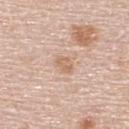A 15 mm close-up tile from a total-body photography series done for melanoma screening.
The lesion is on the upper back.
The lesion-visualizer software estimated radial color variation of about 0.5. The software also gave a classifier nevus-likeness of about 0/100 and a lesion-detection confidence of about 100/100.
Longest diameter approximately 2.5 mm.
This is a white-light tile.
A male patient, aged 58 to 62.